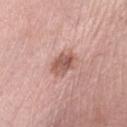  image:
    source: total-body photography crop
    field_of_view_mm: 15
  automated_metrics:
    cielab_L: 57
    cielab_a: 22
    cielab_b: 26
    vs_skin_darker_L: 12.0
    vs_skin_contrast_norm: 8.0
    nevus_likeness_0_100: 20
    lesion_detection_confidence_0_100: 100
  lighting: white-light
  lesion_size:
    long_diameter_mm_approx: 3.5
  patient:
    sex: female
    age_approx: 65
  site: left lower leg